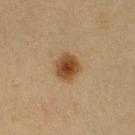{
  "biopsy_status": "not biopsied; imaged during a skin examination",
  "patient": {
    "sex": "male",
    "age_approx": 55
  },
  "site": "right upper arm",
  "image": {
    "source": "total-body photography crop",
    "field_of_view_mm": 15
  }
}